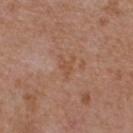{
  "biopsy_status": "not biopsied; imaged during a skin examination",
  "site": "chest",
  "patient": {
    "sex": "male",
    "age_approx": 55
  },
  "image": {
    "source": "total-body photography crop",
    "field_of_view_mm": 15
  },
  "lesion_size": {
    "long_diameter_mm_approx": 3.0
  }
}